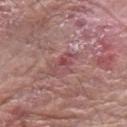<lesion>
  <biopsy_status>not biopsied; imaged during a skin examination</biopsy_status>
  <lesion_size>
    <long_diameter_mm_approx>3.5</long_diameter_mm_approx>
  </lesion_size>
  <lighting>white-light</lighting>
  <patient>
    <sex>male</sex>
    <age_approx>60</age_approx>
  </patient>
  <image>
    <source>total-body photography crop</source>
    <field_of_view_mm>15</field_of_view_mm>
  </image>
  <site>left forearm</site>
</lesion>